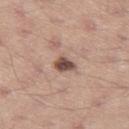{"biopsy_status": "not biopsied; imaged during a skin examination", "image": {"source": "total-body photography crop", "field_of_view_mm": 15}, "patient": {"sex": "male", "age_approx": 35}, "site": "left thigh"}